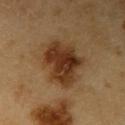The lesion is on the right upper arm. The lesion-visualizer software estimated a border-irregularity rating of about 3/10 and a within-lesion color-variation index near 6/10. Cropped from a total-body skin-imaging series; the visible field is about 15 mm. A male patient approximately 60 years of age.